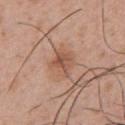<record>
  <biopsy_status>not biopsied; imaged during a skin examination</biopsy_status>
  <site>chest</site>
  <patient>
    <sex>male</sex>
    <age_approx>30</age_approx>
  </patient>
  <lighting>white-light</lighting>
  <image>
    <source>total-body photography crop</source>
    <field_of_view_mm>15</field_of_view_mm>
  </image>
  <lesion_size>
    <long_diameter_mm_approx>3.0</long_diameter_mm_approx>
  </lesion_size>
</record>